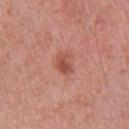Part of a total-body skin-imaging series; this lesion was reviewed on a skin check and was not flagged for biopsy. The lesion is located on the chest. The patient is a male aged around 50. A region of skin cropped from a whole-body photographic capture, roughly 15 mm wide.